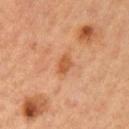Captured during whole-body skin photography for melanoma surveillance; the lesion was not biopsied. A female patient, roughly 40 years of age. The tile uses cross-polarized illumination. A close-up tile cropped from a whole-body skin photograph, about 15 mm across. The recorded lesion diameter is about 2.5 mm. The lesion is on the arm. The total-body-photography lesion software estimated a footprint of about 3 mm² and an outline eccentricity of about 0.85 (0 = round, 1 = elongated). And it measured radial color variation of about 0.5.